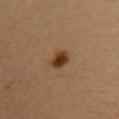Part of a total-body skin-imaging series; this lesion was reviewed on a skin check and was not flagged for biopsy. The tile uses cross-polarized illumination. Located on the front of the torso. Automated tile analysis of the lesion measured a mean CIELAB color near L≈37 a*≈20 b*≈32 and a normalized border contrast of about 11.5. The analysis additionally found border irregularity of about 2 on a 0–10 scale, a color-variation rating of about 3.5/10, and a peripheral color-asymmetry measure near 1. A female subject aged 33 to 37. A roughly 15 mm field-of-view crop from a total-body skin photograph.